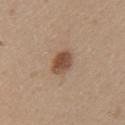follow-up = imaged on a skin check; not biopsied | body site = the left upper arm | automated metrics = a footprint of about 6 mm², a shape eccentricity near 0.6, and two-axis asymmetry of about 0.15; a within-lesion color-variation index near 3/10 and a peripheral color-asymmetry measure near 1 | patient = female, aged 23 to 27 | image = ~15 mm tile from a whole-body skin photo | illumination = white-light illumination.